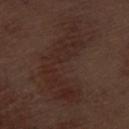Q: Was a biopsy performed?
A: catalogued during a skin exam; not biopsied
Q: What is the imaging modality?
A: total-body-photography crop, ~15 mm field of view
Q: What is the anatomic site?
A: the left thigh
Q: Patient demographics?
A: male, approximately 70 years of age